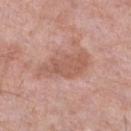| key | value |
|---|---|
| image source | total-body-photography crop, ~15 mm field of view |
| location | the right lower leg |
| patient | male, in their mid-70s |